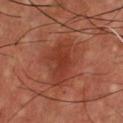Assessment:
Recorded during total-body skin imaging; not selected for excision or biopsy.
Background:
The lesion is located on the chest. Measured at roughly 6 mm in maximum diameter. This is a cross-polarized tile. The subject is a male aged approximately 55. A lesion tile, about 15 mm wide, cut from a 3D total-body photograph.